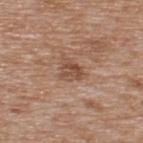notes — total-body-photography surveillance lesion; no biopsy | automated lesion analysis — an eccentricity of roughly 0.5 and two-axis asymmetry of about 0.35; border irregularity of about 3.5 on a 0–10 scale and a color-variation rating of about 4/10; a classifier nevus-likeness of about 0/100 and a detector confidence of about 100 out of 100 that the crop contains a lesion | body site — the upper back | subject — male, aged 63 to 67 | acquisition — ~15 mm crop, total-body skin-cancer survey | lesion diameter — ~3 mm (longest diameter).